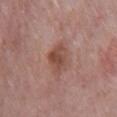This lesion was catalogued during total-body skin photography and was not selected for biopsy. Longest diameter approximately 3.5 mm. The lesion is located on the chest. The subject is a male approximately 65 years of age. Captured under white-light illumination. A region of skin cropped from a whole-body photographic capture, roughly 15 mm wide.